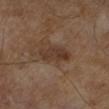  biopsy_status: not biopsied; imaged during a skin examination
  automated_metrics:
    lesion_detection_confidence_0_100: 100
  lighting: cross-polarized
  lesion_size:
    long_diameter_mm_approx: 4.0
  image:
    source: total-body photography crop
    field_of_view_mm: 15
  patient:
    sex: male
    age_approx: 65